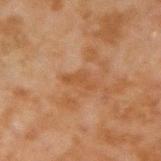* workup — catalogued during a skin exam; not biopsied
* lighting — cross-polarized illumination
* size — ≈3.5 mm
* image source — ~15 mm crop, total-body skin-cancer survey
* site — the right forearm
* patient — male, aged 43–47